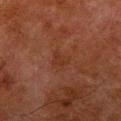| key | value |
|---|---|
| follow-up | imaged on a skin check; not biopsied |
| patient | male, about 80 years old |
| illumination | cross-polarized |
| automated lesion analysis | an area of roughly 4.5 mm², an eccentricity of roughly 0.65, and two-axis asymmetry of about 0.3; a lesion color around L≈26 a*≈20 b*≈25 in CIELAB, a lesion–skin lightness drop of about 4, and a normalized lesion–skin contrast near 5; an automated nevus-likeness rating near 0 out of 100 and a detector confidence of about 100 out of 100 that the crop contains a lesion |
| acquisition | 15 mm crop, total-body photography |
| size | ≈3 mm |
| anatomic site | the right lower leg |